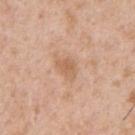Q: Is there a histopathology result?
A: no biopsy performed (imaged during a skin exam)
Q: How was the tile lit?
A: white-light illumination
Q: Patient demographics?
A: male, in their mid- to late 50s
Q: How large is the lesion?
A: about 3 mm
Q: What kind of image is this?
A: total-body-photography crop, ~15 mm field of view
Q: What is the anatomic site?
A: the left upper arm